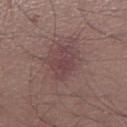Impression: The lesion was tiled from a total-body skin photograph and was not biopsied. Background: A 15 mm close-up tile from a total-body photography series done for melanoma screening. This is a white-light tile. From the left lower leg. Measured at roughly 4 mm in maximum diameter. A male patient roughly 55 years of age.